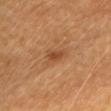Part of a total-body skin-imaging series; this lesion was reviewed on a skin check and was not flagged for biopsy. A female patient approximately 70 years of age. A 15 mm close-up tile from a total-body photography series done for melanoma screening. The lesion is on the chest.